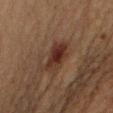<lesion>
<biopsy_status>not biopsied; imaged during a skin examination</biopsy_status>
<image>
  <source>total-body photography crop</source>
  <field_of_view_mm>15</field_of_view_mm>
</image>
<lighting>cross-polarized</lighting>
<patient>
  <sex>male</sex>
  <age_approx>50</age_approx>
</patient>
<site>right upper arm</site>
</lesion>